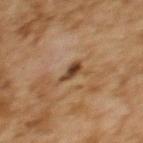A 15 mm close-up tile from a total-body photography series done for melanoma screening. Located on the upper back. A female patient aged around 60.